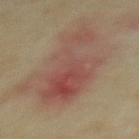Notes:
• follow-up · imaged on a skin check; not biopsied
• anatomic site · the upper back
• diameter · ~8.5 mm (longest diameter)
• illumination · cross-polarized illumination
• automated lesion analysis · an area of roughly 32 mm², an outline eccentricity of about 0.8 (0 = round, 1 = elongated), and a symmetry-axis asymmetry near 0.4
• image · 15 mm crop, total-body photography
• subject · female, aged 33–37